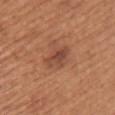biopsy status — catalogued during a skin exam; not biopsied
imaging modality — ~15 mm crop, total-body skin-cancer survey
patient — female, aged 58–62
automated lesion analysis — a border-irregularity rating of about 3/10, a within-lesion color-variation index near 3.5/10, and a peripheral color-asymmetry measure near 1
lighting — white-light illumination
lesion diameter — ~3.5 mm (longest diameter)
location — the right upper arm